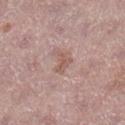{"biopsy_status": "not biopsied; imaged during a skin examination", "image": {"source": "total-body photography crop", "field_of_view_mm": 15}, "lesion_size": {"long_diameter_mm_approx": 2.5}, "site": "right lower leg", "patient": {"sex": "female", "age_approx": 40}}